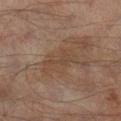biopsy status: total-body-photography surveillance lesion; no biopsy | lesion size: ≈4.5 mm | image-analysis metrics: an eccentricity of roughly 0.9 and two-axis asymmetry of about 0.4; a mean CIELAB color near L≈42 a*≈15 b*≈27 and a lesion-to-skin contrast of about 5 (normalized; higher = more distinct) | location: the right lower leg | image: ~15 mm crop, total-body skin-cancer survey | patient: male, about 65 years old.